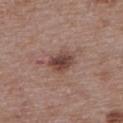workup: total-body-photography surveillance lesion; no biopsy | diameter: ≈3.5 mm | image-analysis metrics: a footprint of about 7 mm², an outline eccentricity of about 0.7 (0 = round, 1 = elongated), and a shape-asymmetry score of about 0.2 (0 = symmetric); radial color variation of about 1.5; an automated nevus-likeness rating near 75 out of 100 | patient: female, aged 63–67 | illumination: white-light | body site: the upper back | imaging modality: ~15 mm crop, total-body skin-cancer survey.